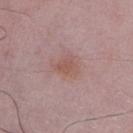{"biopsy_status": "not biopsied; imaged during a skin examination", "site": "right lower leg", "image": {"source": "total-body photography crop", "field_of_view_mm": 15}, "patient": {"sex": "male", "age_approx": 50}}